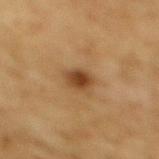Acquisition and patient details:
The patient is a male about 85 years old or older. A lesion tile, about 15 mm wide, cut from a 3D total-body photograph. Measured at roughly 3 mm in maximum diameter. On the mid back. This is a cross-polarized tile.